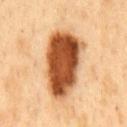Notes:
- follow-up — no biopsy performed (imaged during a skin exam)